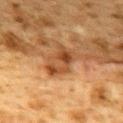<record>
  <biopsy_status>not biopsied; imaged during a skin examination</biopsy_status>
  <lesion_size>
    <long_diameter_mm_approx>4.0</long_diameter_mm_approx>
  </lesion_size>
  <site>upper back</site>
  <patient>
    <sex>female</sex>
    <age_approx>40</age_approx>
  </patient>
  <image>
    <source>total-body photography crop</source>
    <field_of_view_mm>15</field_of_view_mm>
  </image>
</record>